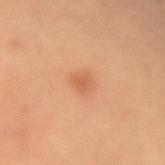No biopsy was performed on this lesion — it was imaged during a full skin examination and was not determined to be concerning. Cropped from a total-body skin-imaging series; the visible field is about 15 mm. The tile uses cross-polarized illumination. The patient is a female aged approximately 65. From the left forearm. The lesion-visualizer software estimated a shape eccentricity near 0.35 and a shape-asymmetry score of about 0.25 (0 = symmetric). And it measured a mean CIELAB color near L≈57 a*≈26 b*≈38, about 8 CIELAB-L* units darker than the surrounding skin, and a lesion-to-skin contrast of about 5.5 (normalized; higher = more distinct). It also reported a nevus-likeness score of about 35/100.